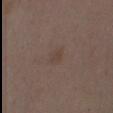Impression: Captured during whole-body skin photography for melanoma surveillance; the lesion was not biopsied. Context: The lesion is located on the front of the torso. A 15 mm close-up tile from a total-body photography series done for melanoma screening. The subject is a female in their mid-30s. Captured under white-light illumination. Measured at roughly 3 mm in maximum diameter.